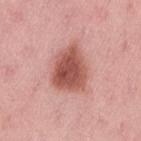Assessment:
Imaged during a routine full-body skin examination; the lesion was not biopsied and no histopathology is available.